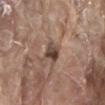Q: Was this lesion biopsied?
A: catalogued during a skin exam; not biopsied
Q: How large is the lesion?
A: ≈3 mm
Q: Patient demographics?
A: male, aged approximately 80
Q: What kind of image is this?
A: ~15 mm tile from a whole-body skin photo
Q: What did automated image analysis measure?
A: an average lesion color of about L≈43 a*≈16 b*≈23 (CIELAB), a lesion–skin lightness drop of about 13, and a lesion-to-skin contrast of about 10 (normalized; higher = more distinct); a border-irregularity index near 2.5/10, internal color variation of about 5 on a 0–10 scale, and a peripheral color-asymmetry measure near 1.5
Q: What is the anatomic site?
A: the back
Q: How was the tile lit?
A: white-light illumination